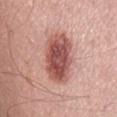Q: Is there a histopathology result?
A: no biopsy performed (imaged during a skin exam)
Q: What is the anatomic site?
A: the mid back
Q: What are the patient's age and sex?
A: male, roughly 65 years of age
Q: What is the imaging modality?
A: ~15 mm crop, total-body skin-cancer survey
Q: How was the tile lit?
A: white-light illumination
Q: Lesion size?
A: ~7 mm (longest diameter)